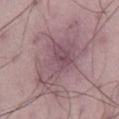  biopsy_status: not biopsied; imaged during a skin examination
  lesion_size:
    long_diameter_mm_approx: 9.0
  image:
    source: total-body photography crop
    field_of_view_mm: 15
  automated_metrics:
    border_irregularity_0_10: 7.0
    color_variation_0_10: 5.0
    peripheral_color_asymmetry: 1.5
    lesion_detection_confidence_0_100: 75
  site: right thigh
  lighting: white-light
  patient:
    sex: male
    age_approx: 50